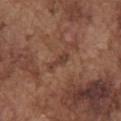No biopsy was performed on this lesion — it was imaged during a full skin examination and was not determined to be concerning.